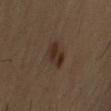Impression:
Recorded during total-body skin imaging; not selected for excision or biopsy.
Context:
Imaged with cross-polarized lighting. A lesion tile, about 15 mm wide, cut from a 3D total-body photograph. The lesion's longest dimension is about 3.5 mm. The subject is a male about 55 years old. On the right upper arm.